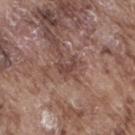follow-up = catalogued during a skin exam; not biopsied | lighting = white-light | acquisition = ~15 mm crop, total-body skin-cancer survey | lesion size = about 2.5 mm | subject = male, aged 73 to 77 | image-analysis metrics = a lesion area of about 3.5 mm², a shape eccentricity near 0.75, and two-axis asymmetry of about 0.45; a border-irregularity index near 5.5/10; a detector confidence of about 55 out of 100 that the crop contains a lesion | anatomic site = the right thigh.